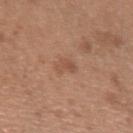<lesion>
<biopsy_status>not biopsied; imaged during a skin examination</biopsy_status>
<site>arm</site>
<patient>
  <sex>female</sex>
  <age_approx>30</age_approx>
</patient>
<image>
  <source>total-body photography crop</source>
  <field_of_view_mm>15</field_of_view_mm>
</image>
</lesion>